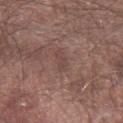No biopsy was performed on this lesion — it was imaged during a full skin examination and was not determined to be concerning. The tile uses white-light illumination. A roughly 15 mm field-of-view crop from a total-body skin photograph. The recorded lesion diameter is about 3 mm. Located on the arm. A male patient in their 80s.